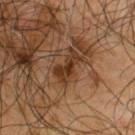biopsy_status: not biopsied; imaged during a skin examination